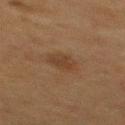Q: Was a biopsy performed?
A: total-body-photography surveillance lesion; no biopsy
Q: Lesion location?
A: the mid back
Q: How was the tile lit?
A: cross-polarized
Q: What kind of image is this?
A: 15 mm crop, total-body photography
Q: Patient demographics?
A: male, roughly 50 years of age